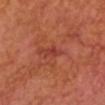follow-up = imaged on a skin check; not biopsied
illumination = cross-polarized illumination
subject = male, aged 63 to 67
image source = ~15 mm crop, total-body skin-cancer survey